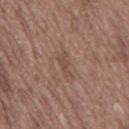Q: Was a biopsy performed?
A: catalogued during a skin exam; not biopsied
Q: What is the lesion's diameter?
A: about 2.5 mm
Q: Who is the patient?
A: male, aged approximately 70
Q: How was the tile lit?
A: white-light illumination
Q: Lesion location?
A: the mid back
Q: What kind of image is this?
A: 15 mm crop, total-body photography